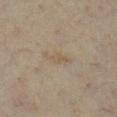Background: The lesion is located on the leg. The recorded lesion diameter is about 3.5 mm. The subject is a female in their mid- to late 60s. A region of skin cropped from a whole-body photographic capture, roughly 15 mm wide. The tile uses cross-polarized illumination.